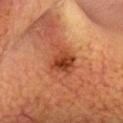follow-up = imaged on a skin check; not biopsied
tile lighting = cross-polarized
acquisition = 15 mm crop, total-body photography
anatomic site = the head or neck
subject = male, aged around 70